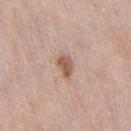automated metrics = a mean CIELAB color near L≈56 a*≈20 b*≈27, roughly 12 lightness units darker than nearby skin, and a normalized border contrast of about 8; illumination = white-light; subject = female, aged 38 to 42; anatomic site = the right thigh; image = total-body-photography crop, ~15 mm field of view.